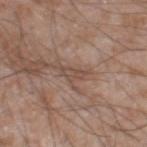follow-up: no biopsy performed (imaged during a skin exam); location: the left thigh; image: ~15 mm tile from a whole-body skin photo; tile lighting: white-light illumination; subject: male, in their 60s.